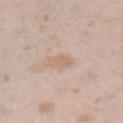Measured at roughly 3 mm in maximum diameter.
This is a white-light tile.
A region of skin cropped from a whole-body photographic capture, roughly 15 mm wide.
The patient is a female aged approximately 25.
From the leg.